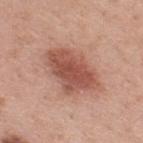Q: Was a biopsy performed?
A: no biopsy performed (imaged during a skin exam)
Q: Patient demographics?
A: male, roughly 40 years of age
Q: What kind of image is this?
A: 15 mm crop, total-body photography
Q: Where on the body is the lesion?
A: the upper back